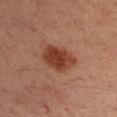<record>
  <biopsy_status>not biopsied; imaged during a skin examination</biopsy_status>
  <site>left upper arm</site>
  <patient>
    <sex>male</sex>
    <age_approx>35</age_approx>
  </patient>
  <image>
    <source>total-body photography crop</source>
    <field_of_view_mm>15</field_of_view_mm>
  </image>
  <lesion_size>
    <long_diameter_mm_approx>4.5</long_diameter_mm_approx>
  </lesion_size>
</record>